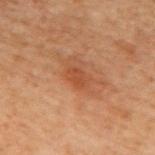<lesion>
<biopsy_status>not biopsied; imaged during a skin examination</biopsy_status>
<lesion_size>
  <long_diameter_mm_approx>2.5</long_diameter_mm_approx>
</lesion_size>
<image>
  <source>total-body photography crop</source>
  <field_of_view_mm>15</field_of_view_mm>
</image>
<site>mid back</site>
<lighting>cross-polarized</lighting>
<patient>
  <sex>male</sex>
  <age_approx>70</age_approx>
</patient>
</lesion>